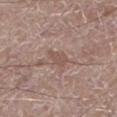biopsy status: catalogued during a skin exam; not biopsied | lighting: white-light illumination | location: the left lower leg | image source: ~15 mm crop, total-body skin-cancer survey | patient: male, aged around 70.